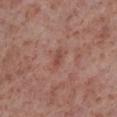workup — imaged on a skin check; not biopsied | body site — the left lower leg | image — 15 mm crop, total-body photography | patient — male, roughly 55 years of age.